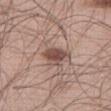<lesion>
<biopsy_status>not biopsied; imaged during a skin examination</biopsy_status>
<lesion_size>
  <long_diameter_mm_approx>3.0</long_diameter_mm_approx>
</lesion_size>
<patient>
  <sex>male</sex>
  <age_approx>60</age_approx>
</patient>
<image>
  <source>total-body photography crop</source>
  <field_of_view_mm>15</field_of_view_mm>
</image>
<site>right thigh</site>
<automated_metrics>
  <cielab_L>49</cielab_L>
  <cielab_a>18</cielab_a>
  <cielab_b>23</cielab_b>
  <vs_skin_darker_L>13.0</vs_skin_darker_L>
  <vs_skin_contrast_norm>9.0</vs_skin_contrast_norm>
  <nevus_likeness_0_100>90</nevus_likeness_0_100>
  <lesion_detection_confidence_0_100>100</lesion_detection_confidence_0_100>
</automated_metrics>
<lighting>white-light</lighting>
</lesion>